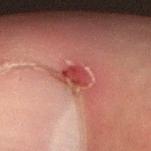Imaged during a routine full-body skin examination; the lesion was not biopsied and no histopathology is available.
The subject is a male about 50 years old.
On the right forearm.
A 15 mm crop from a total-body photograph taken for skin-cancer surveillance.